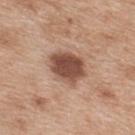Imaged during a routine full-body skin examination; the lesion was not biopsied and no histopathology is available. A female subject aged 38 to 42. Captured under white-light illumination. The lesion is located on the upper back. A lesion tile, about 15 mm wide, cut from a 3D total-body photograph.